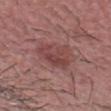Q: Patient demographics?
A: male, approximately 30 years of age
Q: How large is the lesion?
A: ≈6 mm
Q: What kind of image is this?
A: ~15 mm tile from a whole-body skin photo
Q: What did automated image analysis measure?
A: an eccentricity of roughly 0.8 and a shape-asymmetry score of about 0.2 (0 = symmetric); a lesion color around L≈44 a*≈24 b*≈21 in CIELAB, roughly 8 lightness units darker than nearby skin, and a lesion-to-skin contrast of about 6 (normalized; higher = more distinct); border irregularity of about 2.5 on a 0–10 scale, a color-variation rating of about 4.5/10, and peripheral color asymmetry of about 1.5; an automated nevus-likeness rating near 5 out of 100
Q: What lighting was used for the tile?
A: white-light